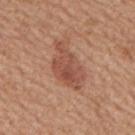follow-up: no biopsy performed (imaged during a skin exam)
anatomic site: the mid back
subject: male, aged 63 to 67
diameter: ~6 mm (longest diameter)
TBP lesion metrics: a lesion area of about 12 mm², an outline eccentricity of about 0.85 (0 = round, 1 = elongated), and two-axis asymmetry of about 0.35; a lesion color around L≈51 a*≈24 b*≈29 in CIELAB and about 10 CIELAB-L* units darker than the surrounding skin
image: total-body-photography crop, ~15 mm field of view